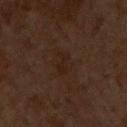Recorded during total-body skin imaging; not selected for excision or biopsy. A male subject, roughly 60 years of age. The lesion is on the front of the torso. This is a cross-polarized tile. Cropped from a whole-body photographic skin survey; the tile spans about 15 mm. About 3 mm across.